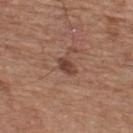Case summary:
- follow-up: catalogued during a skin exam; not biopsied
- image source: ~15 mm crop, total-body skin-cancer survey
- anatomic site: the upper back
- subject: male, aged approximately 65